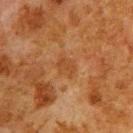Clinical summary: On the upper back. A male patient, aged 78 to 82. A 15 mm close-up extracted from a 3D total-body photography capture. An algorithmic analysis of the crop reported an eccentricity of roughly 0.7 and a symmetry-axis asymmetry near 0.3.